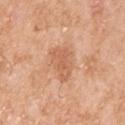Findings:
– workup — no biopsy performed (imaged during a skin exam)
– lesion diameter — about 4 mm
– lighting — white-light illumination
– acquisition — 15 mm crop, total-body photography
– subject — male, in their mid-50s
– automated metrics — a classifier nevus-likeness of about 0/100 and lesion-presence confidence of about 100/100
– body site — the arm